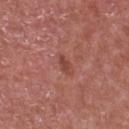The lesion was tiled from a total-body skin photograph and was not biopsied. This is a white-light tile. A 15 mm close-up tile from a total-body photography series done for melanoma screening. The lesion is located on the chest. A male patient aged 63–67. Approximately 2.5 mm at its widest. The lesion-visualizer software estimated a lesion color around L≈44 a*≈27 b*≈27 in CIELAB, about 9 CIELAB-L* units darker than the surrounding skin, and a normalized lesion–skin contrast near 6.5.